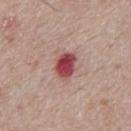Q: Was this lesion biopsied?
A: no biopsy performed (imaged during a skin exam)
Q: Patient demographics?
A: male, aged around 65
Q: What lighting was used for the tile?
A: white-light
Q: What did automated image analysis measure?
A: an area of roughly 6 mm² and an eccentricity of roughly 0.7
Q: What kind of image is this?
A: ~15 mm crop, total-body skin-cancer survey
Q: Lesion location?
A: the abdomen
Q: Lesion size?
A: ~3 mm (longest diameter)